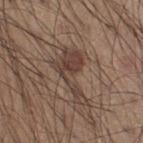The lesion was tiled from a total-body skin photograph and was not biopsied.
A lesion tile, about 15 mm wide, cut from a 3D total-body photograph.
The tile uses white-light illumination.
The lesion is on the leg.
A male patient, aged 53–57.